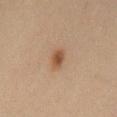Q: Was this lesion biopsied?
A: catalogued during a skin exam; not biopsied
Q: What did automated image analysis measure?
A: a footprint of about 3.5 mm²; a mean CIELAB color near L≈46 a*≈18 b*≈31, about 10 CIELAB-L* units darker than the surrounding skin, and a lesion-to-skin contrast of about 8.5 (normalized; higher = more distinct); a border-irregularity index near 1.5/10, internal color variation of about 3 on a 0–10 scale, and radial color variation of about 1; a classifier nevus-likeness of about 95/100 and a lesion-detection confidence of about 100/100
Q: What are the patient's age and sex?
A: male, approximately 60 years of age
Q: Lesion size?
A: ≈2.5 mm
Q: What is the anatomic site?
A: the abdomen
Q: What lighting was used for the tile?
A: cross-polarized
Q: How was this image acquired?
A: ~15 mm crop, total-body skin-cancer survey